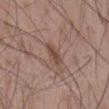{"biopsy_status": "not biopsied; imaged during a skin examination", "image": {"source": "total-body photography crop", "field_of_view_mm": 15}, "patient": {"sex": "male", "age_approx": 55}, "site": "mid back"}